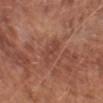Q: Is there a histopathology result?
A: no biopsy performed (imaged during a skin exam)
Q: Patient demographics?
A: male, roughly 65 years of age
Q: What is the anatomic site?
A: the abdomen
Q: What kind of image is this?
A: ~15 mm tile from a whole-body skin photo
Q: Illumination type?
A: white-light illumination